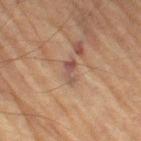No biopsy was performed on this lesion — it was imaged during a full skin examination and was not determined to be concerning. A male subject, approximately 85 years of age or older. Longest diameter approximately 3 mm. Automated image analysis of the tile measured an outline eccentricity of about 0.9 (0 = round, 1 = elongated) and a symmetry-axis asymmetry near 0.4. And it measured about 8 CIELAB-L* units darker than the surrounding skin. The analysis additionally found a border-irregularity rating of about 4.5/10, internal color variation of about 1 on a 0–10 scale, and radial color variation of about 0. And it measured a nevus-likeness score of about 0/100 and lesion-presence confidence of about 75/100. The tile uses cross-polarized illumination. On the left thigh. A 15 mm close-up tile from a total-body photography series done for melanoma screening.